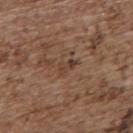Q: Was a biopsy performed?
A: total-body-photography surveillance lesion; no biopsy
Q: What are the patient's age and sex?
A: female, in their mid-60s
Q: What kind of image is this?
A: ~15 mm crop, total-body skin-cancer survey
Q: What is the anatomic site?
A: the back